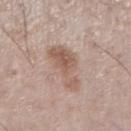Q: Is there a histopathology result?
A: imaged on a skin check; not biopsied
Q: Illumination type?
A: white-light
Q: What is the anatomic site?
A: the right lower leg
Q: What is the imaging modality?
A: ~15 mm crop, total-body skin-cancer survey
Q: Patient demographics?
A: male, roughly 60 years of age
Q: What did automated image analysis measure?
A: an area of roughly 12 mm², an outline eccentricity of about 0.9 (0 = round, 1 = elongated), and two-axis asymmetry of about 0.4; an average lesion color of about L≈57 a*≈18 b*≈26 (CIELAB), a lesion–skin lightness drop of about 10, and a normalized lesion–skin contrast near 7; internal color variation of about 3.5 on a 0–10 scale and radial color variation of about 1; a classifier nevus-likeness of about 40/100 and a detector confidence of about 100 out of 100 that the crop contains a lesion